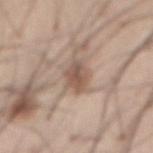Recorded during total-body skin imaging; not selected for excision or biopsy.
A close-up tile cropped from a whole-body skin photograph, about 15 mm across.
Imaged with white-light lighting.
About 4.5 mm across.
A male subject aged approximately 55.
On the abdomen.